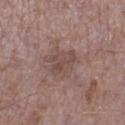{
  "biopsy_status": "not biopsied; imaged during a skin examination",
  "image": {
    "source": "total-body photography crop",
    "field_of_view_mm": 15
  },
  "patient": {
    "sex": "male",
    "age_approx": 70
  },
  "site": "right lower leg"
}